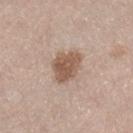– follow-up — total-body-photography surveillance lesion; no biopsy
– body site — the right lower leg
– diameter — about 4 mm
– automated metrics — a border-irregularity index near 2.5/10 and radial color variation of about 1
– image source — ~15 mm crop, total-body skin-cancer survey
– tile lighting — white-light
– subject — female, aged 38 to 42